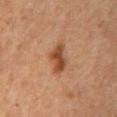Q: What did automated image analysis measure?
A: a lesion area of about 7 mm² and a shape eccentricity near 0.85; a mean CIELAB color near L≈38 a*≈19 b*≈29, about 10 CIELAB-L* units darker than the surrounding skin, and a lesion-to-skin contrast of about 8.5 (normalized; higher = more distinct); border irregularity of about 3 on a 0–10 scale, a within-lesion color-variation index near 3/10, and radial color variation of about 1; a classifier nevus-likeness of about 90/100
Q: Illumination type?
A: cross-polarized illumination
Q: What kind of image is this?
A: ~15 mm tile from a whole-body skin photo
Q: Where on the body is the lesion?
A: the chest
Q: Who is the patient?
A: male, aged approximately 50